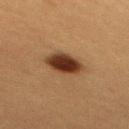Recorded during total-body skin imaging; not selected for excision or biopsy. A 15 mm close-up tile from a total-body photography series done for melanoma screening. A female patient, aged 53 to 57. About 4 mm across. Automated tile analysis of the lesion measured a footprint of about 10 mm². The analysis additionally found border irregularity of about 1.5 on a 0–10 scale, internal color variation of about 4.5 on a 0–10 scale, and peripheral color asymmetry of about 1. And it measured a nevus-likeness score of about 100/100 and a detector confidence of about 100 out of 100 that the crop contains a lesion. Imaged with cross-polarized lighting. On the mid back.